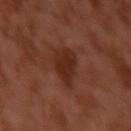Imaged during a routine full-body skin examination; the lesion was not biopsied and no histopathology is available.
Imaged with cross-polarized lighting.
The recorded lesion diameter is about 4 mm.
The lesion is located on the left upper arm.
A male subject, aged 28 to 32.
Cropped from a whole-body photographic skin survey; the tile spans about 15 mm.